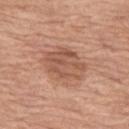Q: Who is the patient?
A: female, in their mid- to late 60s
Q: Automated lesion metrics?
A: a border-irregularity index near 2.5/10, a color-variation rating of about 4.5/10, and radial color variation of about 1.5
Q: How large is the lesion?
A: ≈5 mm
Q: Where on the body is the lesion?
A: the abdomen
Q: How was the tile lit?
A: white-light illumination
Q: How was this image acquired?
A: 15 mm crop, total-body photography